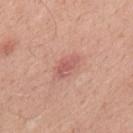biopsy status = catalogued during a skin exam; not biopsied
lesion diameter = ≈3.5 mm
location = the upper back
patient = male, aged 48–52
imaging modality = ~15 mm crop, total-body skin-cancer survey
tile lighting = white-light